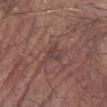{"biopsy_status": "not biopsied; imaged during a skin examination", "automated_metrics": {"area_mm2_approx": 4.0, "eccentricity": 0.7, "shape_asymmetry": 0.4, "cielab_L": 41, "cielab_a": 19, "cielab_b": 20, "vs_skin_darker_L": 7.0, "vs_skin_contrast_norm": 5.5, "border_irregularity_0_10": 4.0, "peripheral_color_asymmetry": 1.0, "nevus_likeness_0_100": 0}, "lighting": "white-light", "patient": {"sex": "male", "age_approx": 80}, "image": {"source": "total-body photography crop", "field_of_view_mm": 15}, "lesion_size": {"long_diameter_mm_approx": 3.0}, "site": "left forearm"}